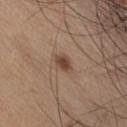Findings:
– automated metrics — an area of roughly 3.5 mm², an outline eccentricity of about 0.75 (0 = round, 1 = elongated), and a symmetry-axis asymmetry near 0.3; a classifier nevus-likeness of about 90/100 and a detector confidence of about 100 out of 100 that the crop contains a lesion
– lesion size — about 2.5 mm
– location — the chest
– patient — male, aged 18 to 22
– image source — ~15 mm tile from a whole-body skin photo